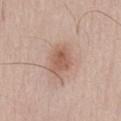About 4 mm across. The lesion is located on the chest. A close-up tile cropped from a whole-body skin photograph, about 15 mm across. A male subject, aged around 65.